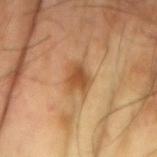Q: Was this lesion biopsied?
A: catalogued during a skin exam; not biopsied
Q: Lesion location?
A: the right arm
Q: Who is the patient?
A: male, aged around 60
Q: How was this image acquired?
A: total-body-photography crop, ~15 mm field of view